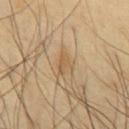The lesion was tiled from a total-body skin photograph and was not biopsied.